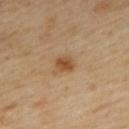No biopsy was performed on this lesion — it was imaged during a full skin examination and was not determined to be concerning.
A female patient, approximately 60 years of age.
The lesion is located on the upper back.
This image is a 15 mm lesion crop taken from a total-body photograph.